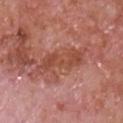Q: What lighting was used for the tile?
A: white-light
Q: Automated lesion metrics?
A: a mean CIELAB color near L≈48 a*≈27 b*≈30, a lesion–skin lightness drop of about 8, and a normalized lesion–skin contrast near 7; a border-irregularity rating of about 5.5/10 and a within-lesion color-variation index near 3.5/10; lesion-presence confidence of about 95/100
Q: What is the lesion's diameter?
A: ≈6.5 mm
Q: Patient demographics?
A: male, aged 63–67
Q: What is the imaging modality?
A: 15 mm crop, total-body photography
Q: What is the anatomic site?
A: the front of the torso